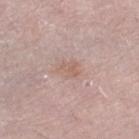Captured during whole-body skin photography for melanoma surveillance; the lesion was not biopsied. A female patient in their mid-60s. Approximately 2.5 mm at its widest. Cropped from a whole-body photographic skin survey; the tile spans about 15 mm. This is a white-light tile. The lesion is located on the left leg.